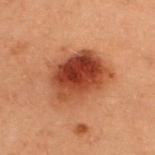| key | value |
|---|---|
| lighting | cross-polarized |
| subject | female, roughly 40 years of age |
| diameter | about 6.5 mm |
| acquisition | total-body-photography crop, ~15 mm field of view |
| location | the upper back |